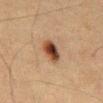Impression:
The lesion was tiled from a total-body skin photograph and was not biopsied.
Background:
A lesion tile, about 15 mm wide, cut from a 3D total-body photograph. The lesion is located on the abdomen. The lesion-visualizer software estimated a mean CIELAB color near L≈35 a*≈18 b*≈26 and roughly 14 lightness units darker than nearby skin. The analysis additionally found a classifier nevus-likeness of about 100/100 and a detector confidence of about 100 out of 100 that the crop contains a lesion. Captured under cross-polarized illumination. Longest diameter approximately 3.5 mm. The subject is a male aged 58–62.